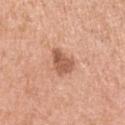Impression:
Imaged during a routine full-body skin examination; the lesion was not biopsied and no histopathology is available.
Clinical summary:
This image is a 15 mm lesion crop taken from a total-body photograph. Captured under white-light illumination. The subject is a female aged 28–32. Located on the left upper arm.